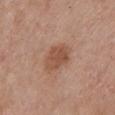Q: Was this lesion biopsied?
A: no biopsy performed (imaged during a skin exam)
Q: Lesion location?
A: the chest
Q: Patient demographics?
A: female, in their mid- to late 60s
Q: How was this image acquired?
A: total-body-photography crop, ~15 mm field of view